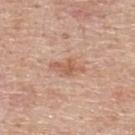Q: Was this lesion biopsied?
A: imaged on a skin check; not biopsied
Q: Where on the body is the lesion?
A: the upper back
Q: What kind of image is this?
A: ~15 mm crop, total-body skin-cancer survey
Q: Patient demographics?
A: male, aged around 55
Q: What did automated image analysis measure?
A: a border-irregularity rating of about 4/10, a color-variation rating of about 2.5/10, and radial color variation of about 1; a classifier nevus-likeness of about 0/100